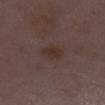notes=no biopsy performed (imaged during a skin exam); patient=female, in their 30s; acquisition=~15 mm tile from a whole-body skin photo; site=the leg.